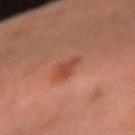<case>
<biopsy_status>not biopsied; imaged during a skin examination</biopsy_status>
<lighting>cross-polarized</lighting>
<patient>
  <sex>male</sex>
  <age_approx>30</age_approx>
</patient>
<site>right forearm</site>
<image>
  <source>total-body photography crop</source>
  <field_of_view_mm>15</field_of_view_mm>
</image>
<automated_metrics>
  <color_variation_0_10>2.5</color_variation_0_10>
</automated_metrics>
<lesion_size>
  <long_diameter_mm_approx>3.0</long_diameter_mm_approx>
</lesion_size>
</case>